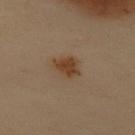This lesion was catalogued during total-body skin photography and was not selected for biopsy.
The lesion is on the back.
An algorithmic analysis of the crop reported a lesion area of about 6.5 mm², an eccentricity of roughly 0.7, and a symmetry-axis asymmetry near 0.3. The analysis additionally found a lesion color around L≈35 a*≈15 b*≈27 in CIELAB and a normalized lesion–skin contrast near 8.5.
The lesion's longest dimension is about 3.5 mm.
A female subject aged 58–62.
This image is a 15 mm lesion crop taken from a total-body photograph.
Captured under cross-polarized illumination.